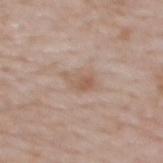Clinical impression: The lesion was tiled from a total-body skin photograph and was not biopsied. Context: The lesion is on the upper back. Imaged with white-light lighting. A region of skin cropped from a whole-body photographic capture, roughly 15 mm wide. About 3.5 mm across. The patient is a female approximately 65 years of age.